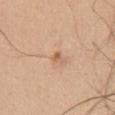| key | value |
|---|---|
| illumination | white-light |
| automated metrics | a lesion color around L≈60 a*≈22 b*≈34 in CIELAB, roughly 10 lightness units darker than nearby skin, and a lesion-to-skin contrast of about 7 (normalized; higher = more distinct); a border-irregularity rating of about 2.5/10, internal color variation of about 0 on a 0–10 scale, and peripheral color asymmetry of about 0 |
| imaging modality | ~15 mm crop, total-body skin-cancer survey |
| location | the chest |
| patient | male, in their mid- to late 30s |